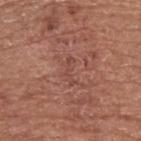Clinical impression:
The lesion was tiled from a total-body skin photograph and was not biopsied.
Clinical summary:
A region of skin cropped from a whole-body photographic capture, roughly 15 mm wide. About 3 mm across. Located on the left upper arm. The tile uses white-light illumination. A male patient, in their mid- to late 60s.